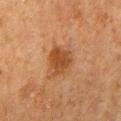This lesion was catalogued during total-body skin photography and was not selected for biopsy.
This image is a 15 mm lesion crop taken from a total-body photograph.
On the lower back.
Automated image analysis of the tile measured a border-irregularity rating of about 3/10, a color-variation rating of about 2.5/10, and a peripheral color-asymmetry measure near 1.
Captured under cross-polarized illumination.
A male subject in their 80s.
The recorded lesion diameter is about 3.5 mm.